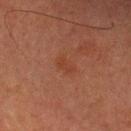biopsy status = no biopsy performed (imaged during a skin exam) | imaging modality = 15 mm crop, total-body photography | anatomic site = the arm | automated lesion analysis = a lesion area of about 3.5 mm² and an eccentricity of roughly 0.8; a border-irregularity index near 3/10, a color-variation rating of about 1.5/10, and radial color variation of about 0.5; a nevus-likeness score of about 0/100 and a lesion-detection confidence of about 100/100 | patient = male, in their 80s | lesion diameter = ≈2.5 mm.